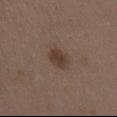| field | value |
|---|---|
| follow-up | imaged on a skin check; not biopsied |
| subject | female, roughly 35 years of age |
| image source | ~15 mm tile from a whole-body skin photo |
| lesion size | about 3.5 mm |
| TBP lesion metrics | a lesion area of about 5.5 mm², a shape eccentricity near 0.8, and a shape-asymmetry score of about 0.15 (0 = symmetric); a nevus-likeness score of about 80/100 and a lesion-detection confidence of about 100/100 |
| lighting | white-light |
| anatomic site | the chest |